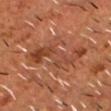Captured during whole-body skin photography for melanoma surveillance; the lesion was not biopsied. This is a cross-polarized tile. Cropped from a total-body skin-imaging series; the visible field is about 15 mm. Longest diameter approximately 6 mm. The lesion is on the head or neck. An algorithmic analysis of the crop reported border irregularity of about 8.5 on a 0–10 scale, a within-lesion color-variation index near 5.5/10, and radial color variation of about 1.5. The patient is a male aged approximately 55.